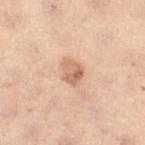Case summary:
• notes — catalogued during a skin exam; not biopsied
• lighting — cross-polarized
• patient — female, aged approximately 55
• image source — total-body-photography crop, ~15 mm field of view
• body site — the abdomen